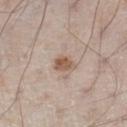The lesion was tiled from a total-body skin photograph and was not biopsied.
From the left lower leg.
A male subject approximately 60 years of age.
Approximately 3 mm at its widest.
Captured under white-light illumination.
A lesion tile, about 15 mm wide, cut from a 3D total-body photograph.